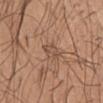Notes:
• follow-up · imaged on a skin check; not biopsied
• TBP lesion metrics · a lesion area of about 4.5 mm², an eccentricity of roughly 0.8, and two-axis asymmetry of about 0.35; a lesion–skin lightness drop of about 7 and a normalized lesion–skin contrast near 5.5; a classifier nevus-likeness of about 0/100 and lesion-presence confidence of about 80/100
• lighting · white-light illumination
• size · about 3.5 mm
• patient · male, roughly 45 years of age
• site · the right upper arm
• image source · total-body-photography crop, ~15 mm field of view